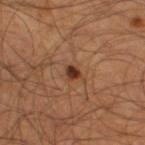Impression:
Captured during whole-body skin photography for melanoma surveillance; the lesion was not biopsied.
Context:
A 15 mm close-up extracted from a 3D total-body photography capture. Imaged with cross-polarized lighting. Located on the right thigh. A male patient aged 53 to 57. Longest diameter approximately 2 mm.